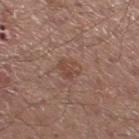  biopsy_status: not biopsied; imaged during a skin examination